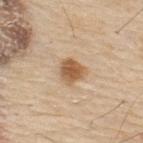biopsy status = catalogued during a skin exam; not biopsied | subject = male, aged 78–82 | location = the upper back | imaging modality = ~15 mm tile from a whole-body skin photo.